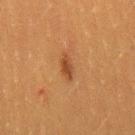{
  "biopsy_status": "not biopsied; imaged during a skin examination",
  "lighting": "cross-polarized",
  "automated_metrics": {
    "nevus_likeness_0_100": 50,
    "lesion_detection_confidence_0_100": 100
  },
  "patient": {
    "sex": "female",
    "age_approx": 40
  },
  "lesion_size": {
    "long_diameter_mm_approx": 3.5
  },
  "image": {
    "source": "total-body photography crop",
    "field_of_view_mm": 15
  },
  "site": "leg"
}